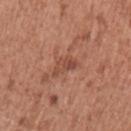Q: Was this lesion biopsied?
A: no biopsy performed (imaged during a skin exam)
Q: Patient demographics?
A: female, approximately 50 years of age
Q: How was this image acquired?
A: total-body-photography crop, ~15 mm field of view
Q: What is the anatomic site?
A: the left upper arm